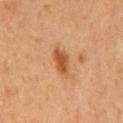Image and clinical context:
The lesion's longest dimension is about 3.5 mm. This is a cross-polarized tile. A 15 mm crop from a total-body photograph taken for skin-cancer surveillance. A male patient, approximately 60 years of age. Located on the chest.